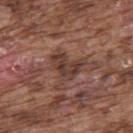biopsy_status: not biopsied; imaged during a skin examination
lighting: white-light
patient:
  sex: male
  age_approx: 75
automated_metrics:
  area_mm2_approx: 9.5
  eccentricity: 0.55
  shape_asymmetry: 0.5
  vs_skin_darker_L: 9.0
  vs_skin_contrast_norm: 8.0
  border_irregularity_0_10: 5.5
  color_variation_0_10: 4.0
  peripheral_color_asymmetry: 1.5
  nevus_likeness_0_100: 5
  lesion_detection_confidence_0_100: 80
site: upper back
image:
  source: total-body photography crop
  field_of_view_mm: 15